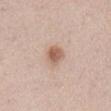Part of a total-body skin-imaging series; this lesion was reviewed on a skin check and was not flagged for biopsy. The tile uses white-light illumination. An algorithmic analysis of the crop reported a color-variation rating of about 3.5/10. It also reported an automated nevus-likeness rating near 95 out of 100 and lesion-presence confidence of about 100/100. A female patient in their mid- to late 60s. A 15 mm close-up tile from a total-body photography series done for melanoma screening. About 2.5 mm across. From the front of the torso.